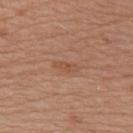Clinical summary:
Located on the upper back. A male subject approximately 80 years of age. The tile uses white-light illumination. A 15 mm close-up tile from a total-body photography series done for melanoma screening. The total-body-photography lesion software estimated roughly 7 lightness units darker than nearby skin and a normalized border contrast of about 6. It also reported a border-irregularity rating of about 3.5/10, internal color variation of about 0 on a 0–10 scale, and a peripheral color-asymmetry measure near 0. And it measured an automated nevus-likeness rating near 0 out of 100 and lesion-presence confidence of about 100/100.